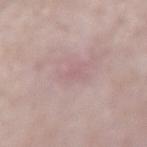image: total-body-photography crop, ~15 mm field of view
location: the right forearm
TBP lesion metrics: a lesion area of about 1 mm² and a shape-asymmetry score of about 0.45 (0 = symmetric); a mean CIELAB color near L≈59 a*≈20 b*≈17, roughly 4 lightness units darker than nearby skin, and a normalized border contrast of about 3; border irregularity of about 3.5 on a 0–10 scale, a within-lesion color-variation index near 0/10, and a peripheral color-asymmetry measure near 0; a classifier nevus-likeness of about 0/100 and a detector confidence of about 75 out of 100 that the crop contains a lesion
size: ~1.5 mm (longest diameter)
illumination: white-light illumination
subject: female, in their 50s
histopathology: a dermatofibroma (benign)